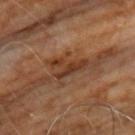Q: Was this lesion biopsied?
A: no biopsy performed (imaged during a skin exam)